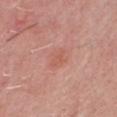No biopsy was performed on this lesion — it was imaged during a full skin examination and was not determined to be concerning. This image is a 15 mm lesion crop taken from a total-body photograph. Located on the front of the torso. The patient is a male approximately 50 years of age.